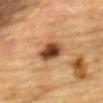Captured during whole-body skin photography for melanoma surveillance; the lesion was not biopsied. A lesion tile, about 15 mm wide, cut from a 3D total-body photograph. The recorded lesion diameter is about 4 mm. The lesion is located on the front of the torso. Imaged with cross-polarized lighting. A male patient, aged around 85 or older.